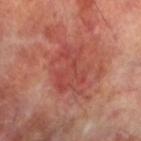biopsy status: total-body-photography surveillance lesion; no biopsy | diameter: ~5.5 mm (longest diameter) | body site: the leg | automated metrics: a mean CIELAB color near L≈46 a*≈30 b*≈28 and roughly 7 lightness units darker than nearby skin | illumination: cross-polarized illumination | image: ~15 mm crop, total-body skin-cancer survey | subject: male, approximately 70 years of age.